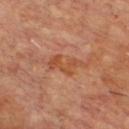Part of a total-body skin-imaging series; this lesion was reviewed on a skin check and was not flagged for biopsy. From the upper back. Captured under cross-polarized illumination. A region of skin cropped from a whole-body photographic capture, roughly 15 mm wide. The subject is a male roughly 65 years of age. The recorded lesion diameter is about 4.5 mm. An algorithmic analysis of the crop reported an average lesion color of about L≈45 a*≈24 b*≈33 (CIELAB) and a normalized lesion–skin contrast near 6.5. And it measured a classifier nevus-likeness of about 0/100 and lesion-presence confidence of about 100/100.